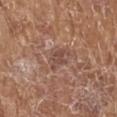subject = female, aged approximately 75; diameter = ≈3 mm; body site = the left lower leg; tile lighting = white-light illumination; acquisition = 15 mm crop, total-body photography.